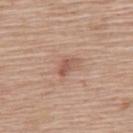<record>
<biopsy_status>not biopsied; imaged during a skin examination</biopsy_status>
<patient>
  <sex>male</sex>
  <age_approx>60</age_approx>
</patient>
<image>
  <source>total-body photography crop</source>
  <field_of_view_mm>15</field_of_view_mm>
</image>
<automated_metrics>
  <cielab_L>54</cielab_L>
  <cielab_a>22</cielab_a>
  <cielab_b>28</cielab_b>
  <vs_skin_contrast_norm>6.5</vs_skin_contrast_norm>
</automated_metrics>
<site>upper back</site>
<lighting>white-light</lighting>
<lesion_size>
  <long_diameter_mm_approx>2.5</long_diameter_mm_approx>
</lesion_size>
</record>